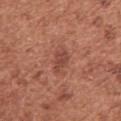| field | value |
|---|---|
| notes | total-body-photography surveillance lesion; no biopsy |
| site | the arm |
| image | ~15 mm tile from a whole-body skin photo |
| tile lighting | white-light |
| diameter | ~3 mm (longest diameter) |
| patient | female, aged 48–52 |